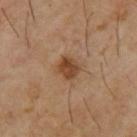The lesion was tiled from a total-body skin photograph and was not biopsied.
On the upper back.
Automated tile analysis of the lesion measured an area of roughly 5.5 mm², a shape eccentricity near 0.4, and a symmetry-axis asymmetry near 0.2. And it measured a mean CIELAB color near L≈41 a*≈19 b*≈31. The software also gave a border-irregularity rating of about 1.5/10 and radial color variation of about 1. And it measured an automated nevus-likeness rating near 90 out of 100 and a lesion-detection confidence of about 100/100.
Captured under cross-polarized illumination.
The subject is a male in their mid- to late 60s.
Cropped from a total-body skin-imaging series; the visible field is about 15 mm.
About 2.5 mm across.